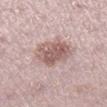Part of a total-body skin-imaging series; this lesion was reviewed on a skin check and was not flagged for biopsy. A region of skin cropped from a whole-body photographic capture, roughly 15 mm wide. The lesion-visualizer software estimated a footprint of about 14 mm², an outline eccentricity of about 0.45 (0 = round, 1 = elongated), and two-axis asymmetry of about 0.15. The analysis additionally found an average lesion color of about L≈58 a*≈19 b*≈21 (CIELAB) and a lesion-to-skin contrast of about 8 (normalized; higher = more distinct). It also reported a classifier nevus-likeness of about 20/100 and lesion-presence confidence of about 100/100. Measured at roughly 4.5 mm in maximum diameter. The lesion is on the right lower leg. The subject is a female in their mid-40s. The tile uses white-light illumination.